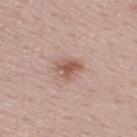{
  "biopsy_status": "not biopsied; imaged during a skin examination",
  "image": {
    "source": "total-body photography crop",
    "field_of_view_mm": 15
  },
  "lighting": "white-light",
  "site": "upper back",
  "patient": {
    "sex": "male",
    "age_approx": 60
  }
}